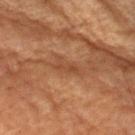lesion diameter — ~3 mm (longest diameter) | automated metrics — a lesion area of about 3 mm² and a shape eccentricity near 0.95; an automated nevus-likeness rating near 0 out of 100 | subject — female, aged around 80 | tile lighting — cross-polarized illumination | imaging modality — ~15 mm tile from a whole-body skin photo | site — the arm.